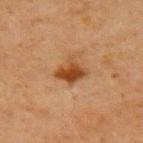Acquisition and patient details: A male patient aged 68 to 72. The lesion's longest dimension is about 3.5 mm. A lesion tile, about 15 mm wide, cut from a 3D total-body photograph. Automated tile analysis of the lesion measured a footprint of about 7 mm², a shape eccentricity near 0.55, and two-axis asymmetry of about 0.25. The lesion is located on the right upper arm.